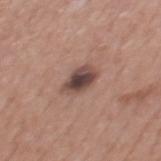This lesion was catalogued during total-body skin photography and was not selected for biopsy. Longest diameter approximately 3.5 mm. A 15 mm crop from a total-body photograph taken for skin-cancer surveillance. The total-body-photography lesion software estimated an area of roughly 7 mm² and an eccentricity of roughly 0.8. The software also gave a mean CIELAB color near L≈45 a*≈18 b*≈21, a lesion–skin lightness drop of about 14, and a lesion-to-skin contrast of about 10.5 (normalized; higher = more distinct). The software also gave a classifier nevus-likeness of about 20/100. A male subject aged around 55. This is a white-light tile. The lesion is located on the mid back.